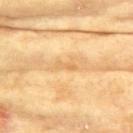Captured during whole-body skin photography for melanoma surveillance; the lesion was not biopsied. The subject is a female aged approximately 75. The lesion is located on the chest. A roughly 15 mm field-of-view crop from a total-body skin photograph.